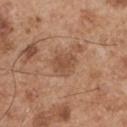This lesion was catalogued during total-body skin photography and was not selected for biopsy. This image is a 15 mm lesion crop taken from a total-body photograph. About 3 mm across. The tile uses white-light illumination. On the right upper arm. A male subject approximately 55 years of age. An algorithmic analysis of the crop reported a shape eccentricity near 0.45 and two-axis asymmetry of about 0.25. The analysis additionally found a lesion color around L≈50 a*≈21 b*≈32 in CIELAB, roughly 8 lightness units darker than nearby skin, and a normalized lesion–skin contrast near 6. The software also gave a nevus-likeness score of about 5/100 and lesion-presence confidence of about 100/100.